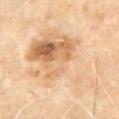Part of a total-body skin-imaging series; this lesion was reviewed on a skin check and was not flagged for biopsy.
The subject is a male approximately 70 years of age.
A lesion tile, about 15 mm wide, cut from a 3D total-body photograph.
Located on the front of the torso.
Approximately 12.5 mm at its widest.
Imaged with cross-polarized lighting.
Automated tile analysis of the lesion measured a border-irregularity index near 7/10.